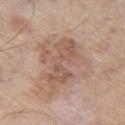{
  "biopsy_status": "not biopsied; imaged during a skin examination",
  "lesion_size": {
    "long_diameter_mm_approx": 6.5
  },
  "site": "left thigh",
  "image": {
    "source": "total-body photography crop",
    "field_of_view_mm": 15
  },
  "lighting": "white-light",
  "patient": {
    "sex": "male",
    "age_approx": 60
  }
}